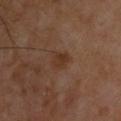Imaged during a routine full-body skin examination; the lesion was not biopsied and no histopathology is available. Imaged with cross-polarized lighting. A male subject, about 55 years old. Cropped from a total-body skin-imaging series; the visible field is about 15 mm. The lesion is located on the upper back. Automated tile analysis of the lesion measured a shape eccentricity near 0.7 and a symmetry-axis asymmetry near 0.2. And it measured a classifier nevus-likeness of about 10/100.